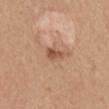Part of a total-body skin-imaging series; this lesion was reviewed on a skin check and was not flagged for biopsy.
Automated image analysis of the tile measured an area of roughly 3.5 mm², an outline eccentricity of about 0.85 (0 = round, 1 = elongated), and two-axis asymmetry of about 0.3. The analysis additionally found an average lesion color of about L≈53 a*≈21 b*≈31 (CIELAB), roughly 11 lightness units darker than nearby skin, and a normalized border contrast of about 7.5.
A female subject aged 33–37.
A 15 mm crop from a total-body photograph taken for skin-cancer surveillance.
The tile uses white-light illumination.
The lesion is located on the mid back.
Longest diameter approximately 3 mm.